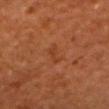Clinical impression:
Captured during whole-body skin photography for melanoma surveillance; the lesion was not biopsied.
Clinical summary:
A 15 mm close-up tile from a total-body photography series done for melanoma screening. The lesion's longest dimension is about 2.5 mm. The lesion is on the front of the torso. The patient is a male roughly 60 years of age.